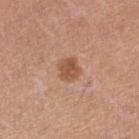This lesion was catalogued during total-body skin photography and was not selected for biopsy.
A 15 mm crop from a total-body photograph taken for skin-cancer surveillance.
A female patient roughly 65 years of age.
On the left lower leg.